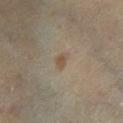Q: Is there a histopathology result?
A: no biopsy performed (imaged during a skin exam)
Q: What lighting was used for the tile?
A: cross-polarized
Q: What kind of image is this?
A: total-body-photography crop, ~15 mm field of view
Q: What is the lesion's diameter?
A: about 2 mm
Q: Who is the patient?
A: female, about 65 years old
Q: What is the anatomic site?
A: the left lower leg